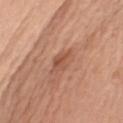Imaged during a routine full-body skin examination; the lesion was not biopsied and no histopathology is available. Captured under white-light illumination. Automated image analysis of the tile measured an average lesion color of about L≈53 a*≈24 b*≈31 (CIELAB), about 9 CIELAB-L* units darker than the surrounding skin, and a normalized lesion–skin contrast near 6. The analysis additionally found a border-irregularity index near 4.5/10, a color-variation rating of about 3.5/10, and peripheral color asymmetry of about 1. The lesion is located on the arm. Approximately 4 mm at its widest. A female subject, aged around 65. Cropped from a total-body skin-imaging series; the visible field is about 15 mm.